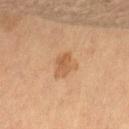| feature | finding |
|---|---|
| biopsy status | catalogued during a skin exam; not biopsied |
| image | ~15 mm crop, total-body skin-cancer survey |
| anatomic site | the leg |
| patient | female, aged 68 to 72 |